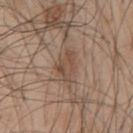Assessment: Part of a total-body skin-imaging series; this lesion was reviewed on a skin check and was not flagged for biopsy.